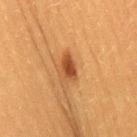  biopsy_status: not biopsied; imaged during a skin examination
  patient:
    sex: female
    age_approx: 40
  lesion_size:
    long_diameter_mm_approx: 3.0
  lighting: cross-polarized
  site: leg
  image:
    source: total-body photography crop
    field_of_view_mm: 15